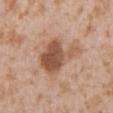Assessment:
No biopsy was performed on this lesion — it was imaged during a full skin examination and was not determined to be concerning.
Context:
The patient is a male aged approximately 45. The lesion is located on the front of the torso. Captured under white-light illumination. The lesion's longest dimension is about 6 mm. A close-up tile cropped from a whole-body skin photograph, about 15 mm across.